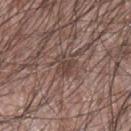biopsy status: imaged on a skin check; not biopsied | lighting: white-light | lesion diameter: ≈2.5 mm | patient: male, about 60 years old | location: the arm | acquisition: ~15 mm tile from a whole-body skin photo.